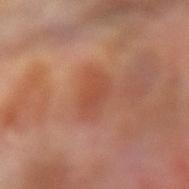Q: Was a biopsy performed?
A: catalogued during a skin exam; not biopsied
Q: How was this image acquired?
A: ~15 mm crop, total-body skin-cancer survey
Q: What is the lesion's diameter?
A: ~4 mm (longest diameter)
Q: Patient demographics?
A: female, approximately 75 years of age
Q: Where on the body is the lesion?
A: the left forearm
Q: What did automated image analysis measure?
A: two-axis asymmetry of about 0.2; an average lesion color of about L≈50 a*≈27 b*≈33 (CIELAB), about 7 CIELAB-L* units darker than the surrounding skin, and a normalized border contrast of about 5; a border-irregularity index near 2.5/10, internal color variation of about 3 on a 0–10 scale, and radial color variation of about 1
Q: How was the tile lit?
A: cross-polarized illumination